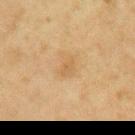• follow-up: catalogued during a skin exam; not biopsied
• imaging modality: ~15 mm crop, total-body skin-cancer survey
• lighting: cross-polarized illumination
• diameter: ≈2.5 mm
• patient: male, about 65 years old
• location: the right upper arm